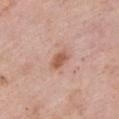Q: Was this lesion biopsied?
A: catalogued during a skin exam; not biopsied
Q: Lesion size?
A: about 2.5 mm
Q: Illumination type?
A: white-light illumination
Q: Patient demographics?
A: female, approximately 65 years of age
Q: What is the anatomic site?
A: the chest
Q: What kind of image is this?
A: 15 mm crop, total-body photography